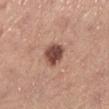Clinical impression: Imaged during a routine full-body skin examination; the lesion was not biopsied and no histopathology is available. Acquisition and patient details: Cropped from a whole-body photographic skin survey; the tile spans about 15 mm. From the left lower leg. The subject is a female approximately 65 years of age.